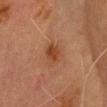Clinical impression:
No biopsy was performed on this lesion — it was imaged during a full skin examination and was not determined to be concerning.
Acquisition and patient details:
A region of skin cropped from a whole-body photographic capture, roughly 15 mm wide. A male subject, aged 68 to 72. The lesion is located on the head or neck. The tile uses cross-polarized illumination. The recorded lesion diameter is about 3.5 mm.